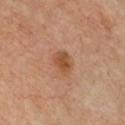biopsy_status: not biopsied; imaged during a skin examination
patient:
  sex: male
  age_approx: 55
image:
  source: total-body photography crop
  field_of_view_mm: 15
site: front of the torso